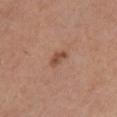Q: Was this lesion biopsied?
A: no biopsy performed (imaged during a skin exam)
Q: What is the imaging modality?
A: total-body-photography crop, ~15 mm field of view
Q: Where on the body is the lesion?
A: the front of the torso
Q: Lesion size?
A: ~3 mm (longest diameter)
Q: Automated lesion metrics?
A: a lesion area of about 3 mm², an eccentricity of roughly 0.85, and two-axis asymmetry of about 0.4; a lesion color around L≈49 a*≈22 b*≈30 in CIELAB, a lesion–skin lightness drop of about 10, and a lesion-to-skin contrast of about 7.5 (normalized; higher = more distinct)
Q: Illumination type?
A: white-light illumination
Q: Patient demographics?
A: female, aged approximately 45